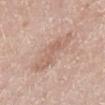Case summary:
• notes: catalogued during a skin exam; not biopsied
• body site: the right lower leg
• patient: female, in their 60s
• imaging modality: ~15 mm tile from a whole-body skin photo